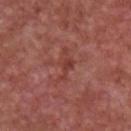Background: Imaged with white-light lighting. A close-up tile cropped from a whole-body skin photograph, about 15 mm across. A male subject, roughly 65 years of age. Located on the chest.